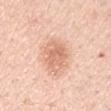Notes:
* biopsy status — catalogued during a skin exam; not biopsied
* image — ~15 mm crop, total-body skin-cancer survey
* subject — male, aged 63–67
* size — about 5 mm
* lighting — white-light
* anatomic site — the arm